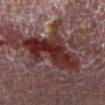Clinical impression: This lesion was catalogued during total-body skin photography and was not selected for biopsy. Clinical summary: A male subject aged 53–57. This image is a 15 mm lesion crop taken from a total-body photograph. Located on the left lower leg. The recorded lesion diameter is about 9 mm. This is a cross-polarized tile.